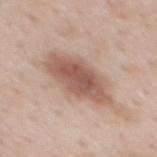Case summary:
• biopsy status — total-body-photography surveillance lesion; no biopsy
• anatomic site — the mid back
• diameter — about 7.5 mm
• illumination — white-light
• automated metrics — a footprint of about 19 mm²
• patient — male, approximately 50 years of age
• acquisition — ~15 mm crop, total-body skin-cancer survey